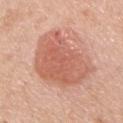follow-up=no biopsy performed (imaged during a skin exam); tile lighting=white-light illumination; location=the chest; patient=male, aged 43 to 47; diameter=≈8.5 mm; acquisition=~15 mm tile from a whole-body skin photo.